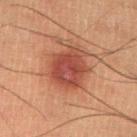Assessment:
The lesion was tiled from a total-body skin photograph and was not biopsied.
Image and clinical context:
On the left lower leg. The lesion's longest dimension is about 4.5 mm. A 15 mm close-up tile from a total-body photography series done for melanoma screening. Automated tile analysis of the lesion measured an eccentricity of roughly 0.35 and two-axis asymmetry of about 0.2. The software also gave a mean CIELAB color near L≈36 a*≈23 b*≈25 and a normalized lesion–skin contrast near 8. The analysis additionally found a nevus-likeness score of about 95/100 and lesion-presence confidence of about 100/100. The tile uses cross-polarized illumination. A male patient approximately 50 years of age.